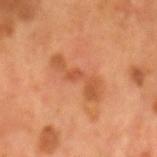{
  "biopsy_status": "not biopsied; imaged during a skin examination",
  "lesion_size": {
    "long_diameter_mm_approx": 6.0
  },
  "lighting": "cross-polarized",
  "automated_metrics": {
    "area_mm2_approx": 9.5,
    "eccentricity": 0.95,
    "shape_asymmetry": 0.45
  },
  "patient": {
    "sex": "male",
    "age_approx": 55
  },
  "image": {
    "source": "total-body photography crop",
    "field_of_view_mm": 15
  },
  "site": "head or neck"
}